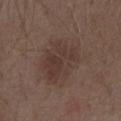Assessment: Recorded during total-body skin imaging; not selected for excision or biopsy. Background: The lesion is located on the abdomen. A male subject aged around 70. Imaged with white-light lighting. This image is a 15 mm lesion crop taken from a total-body photograph. About 6.5 mm across. Automated tile analysis of the lesion measured lesion-presence confidence of about 100/100.